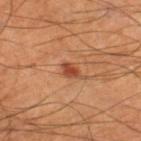- biopsy status · catalogued during a skin exam; not biopsied
- image-analysis metrics · a lesion area of about 3 mm² and a symmetry-axis asymmetry near 0.25
- patient · male, aged approximately 65
- lesion diameter · ~2.5 mm (longest diameter)
- body site · the leg
- image source · 15 mm crop, total-body photography